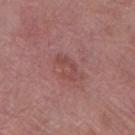Q: Was this lesion biopsied?
A: no biopsy performed (imaged during a skin exam)
Q: Patient demographics?
A: female, aged 68 to 72
Q: Lesion location?
A: the left thigh
Q: What lighting was used for the tile?
A: white-light illumination
Q: What is the imaging modality?
A: 15 mm crop, total-body photography
Q: What did automated image analysis measure?
A: about 7 CIELAB-L* units darker than the surrounding skin and a normalized border contrast of about 5.5; a border-irregularity rating of about 9.5/10, a within-lesion color-variation index near 0/10, and a peripheral color-asymmetry measure near 0; a nevus-likeness score of about 0/100 and a lesion-detection confidence of about 100/100